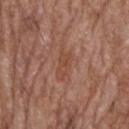Clinical impression: This lesion was catalogued during total-body skin photography and was not selected for biopsy. Image and clinical context: A 15 mm close-up extracted from a 3D total-body photography capture. A male subject, aged 68 to 72. An algorithmic analysis of the crop reported a border-irregularity index near 3/10, internal color variation of about 3 on a 0–10 scale, and a peripheral color-asymmetry measure near 1. Approximately 3 mm at its widest. From the upper back.